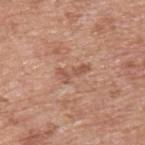• diameter: ≈3.5 mm
• subject: male, aged approximately 55
• illumination: white-light illumination
• site: the back
• TBP lesion metrics: a lesion area of about 4 mm², an outline eccentricity of about 0.9 (0 = round, 1 = elongated), and two-axis asymmetry of about 0.5; a border-irregularity rating of about 6.5/10, a color-variation rating of about 0/10, and peripheral color asymmetry of about 0; an automated nevus-likeness rating near 0 out of 100 and a detector confidence of about 100 out of 100 that the crop contains a lesion
• image: 15 mm crop, total-body photography